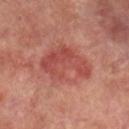Recorded during total-body skin imaging; not selected for excision or biopsy.
This is a cross-polarized tile.
This image is a 15 mm lesion crop taken from a total-body photograph.
A male patient, aged approximately 70.
Located on the right lower leg.